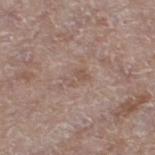No biopsy was performed on this lesion — it was imaged during a full skin examination and was not determined to be concerning. A close-up tile cropped from a whole-body skin photograph, about 15 mm across. The lesion is on the left thigh. The tile uses white-light illumination. Automated image analysis of the tile measured an area of roughly 3.5 mm², an eccentricity of roughly 0.8, and a symmetry-axis asymmetry near 0.35. The analysis additionally found a color-variation rating of about 1/10 and a peripheral color-asymmetry measure near 0. A female patient, aged 73–77. The lesion's longest dimension is about 3 mm.